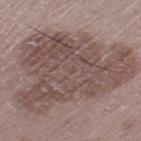Captured during whole-body skin photography for melanoma surveillance; the lesion was not biopsied.
About 13 mm across.
A male subject in their mid- to late 50s.
The lesion is on the leg.
This is a white-light tile.
A lesion tile, about 15 mm wide, cut from a 3D total-body photograph.